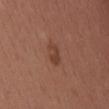A 15 mm close-up extracted from a 3D total-body photography capture. Imaged with white-light lighting. On the head or neck. A male subject, about 40 years old.